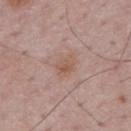  biopsy_status: not biopsied; imaged during a skin examination
  lighting: white-light
  lesion_size:
    long_diameter_mm_approx: 3.0
  image:
    source: total-body photography crop
    field_of_view_mm: 15
  automated_metrics:
    area_mm2_approx: 4.5
    eccentricity: 0.75
    shape_asymmetry: 0.25
    border_irregularity_0_10: 2.5
    peripheral_color_asymmetry: 1.0
    nevus_likeness_0_100: 5
    lesion_detection_confidence_0_100: 100
  patient:
    sex: male
    age_approx: 50
  site: back